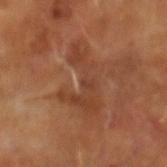Imaged during a routine full-body skin examination; the lesion was not biopsied and no histopathology is available.
Automated image analysis of the tile measured an outline eccentricity of about 0.85 (0 = round, 1 = elongated) and a shape-asymmetry score of about 0.6 (0 = symmetric). The analysis additionally found a border-irregularity rating of about 9.5/10, a color-variation rating of about 2.5/10, and a peripheral color-asymmetry measure near 0.5.
A roughly 15 mm field-of-view crop from a total-body skin photograph.
This is a cross-polarized tile.
The recorded lesion diameter is about 7 mm.
The patient is a male roughly 65 years of age.